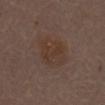Recorded during total-body skin imaging; not selected for excision or biopsy.